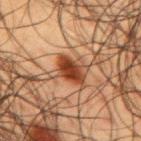Clinical impression:
The lesion was photographed on a routine skin check and not biopsied; there is no pathology result.
Image and clinical context:
The lesion is located on the upper back. A male subject, aged approximately 60. A 15 mm crop from a total-body photograph taken for skin-cancer surveillance. Automated image analysis of the tile measured a lesion area of about 5.5 mm², an eccentricity of roughly 0.85, and two-axis asymmetry of about 0.25. It also reported a border-irregularity rating of about 2/10, internal color variation of about 2.5 on a 0–10 scale, and radial color variation of about 1.